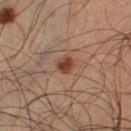Case summary:
- follow-up · imaged on a skin check; not biopsied
- illumination · cross-polarized
- image · total-body-photography crop, ~15 mm field of view
- TBP lesion metrics · an eccentricity of roughly 0.5 and two-axis asymmetry of about 0.2; a lesion color around L≈40 a*≈21 b*≈29 in CIELAB, roughly 11 lightness units darker than nearby skin, and a lesion-to-skin contrast of about 9.5 (normalized; higher = more distinct); a classifier nevus-likeness of about 95/100
- patient · male, in their mid- to late 60s
- body site · the left thigh
- size · ≈2 mm